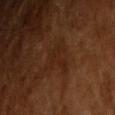workup = imaged on a skin check; not biopsied
lesion size = ~4 mm (longest diameter)
subject = male, roughly 65 years of age
imaging modality = total-body-photography crop, ~15 mm field of view